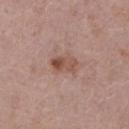biopsy_status: not biopsied; imaged during a skin examination
patient:
  sex: female
  age_approx: 65
image:
  source: total-body photography crop
  field_of_view_mm: 15
lighting: white-light
lesion_size:
  long_diameter_mm_approx: 3.5
site: right lower leg